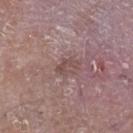workup: total-body-photography surveillance lesion; no biopsy
image: ~15 mm tile from a whole-body skin photo
location: the right lower leg
subject: female, in their mid- to late 70s
illumination: white-light